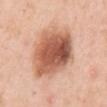A roughly 15 mm field-of-view crop from a total-body skin photograph.
The lesion's longest dimension is about 8 mm.
The lesion is located on the right upper arm.
The patient is a female approximately 50 years of age.
Automated image analysis of the tile measured a lesion color around L≈58 a*≈25 b*≈32 in CIELAB, a lesion–skin lightness drop of about 17, and a lesion-to-skin contrast of about 10.5 (normalized; higher = more distinct). The analysis additionally found a color-variation rating of about 8.5/10. The software also gave a classifier nevus-likeness of about 90/100.
Imaged with white-light lighting.